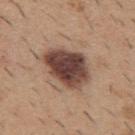Q: Is there a histopathology result?
A: catalogued during a skin exam; not biopsied
Q: What did automated image analysis measure?
A: border irregularity of about 2 on a 0–10 scale and a color-variation rating of about 6/10; a nevus-likeness score of about 65/100
Q: What is the lesion's diameter?
A: ~5.5 mm (longest diameter)
Q: What is the anatomic site?
A: the mid back
Q: What are the patient's age and sex?
A: male, aged 38 to 42
Q: How was the tile lit?
A: white-light
Q: How was this image acquired?
A: 15 mm crop, total-body photography